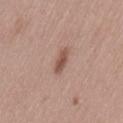{
  "biopsy_status": "not biopsied; imaged during a skin examination",
  "site": "left thigh",
  "patient": {
    "sex": "female",
    "age_approx": 40
  },
  "image": {
    "source": "total-body photography crop",
    "field_of_view_mm": 15
  }
}